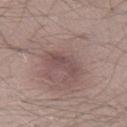The lesion was tiled from a total-body skin photograph and was not biopsied.
Cropped from a whole-body photographic skin survey; the tile spans about 15 mm.
The lesion is on the right thigh.
A male subject aged 23–27.